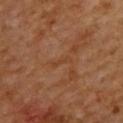This lesion was catalogued during total-body skin photography and was not selected for biopsy. This is a cross-polarized tile. Cropped from a total-body skin-imaging series; the visible field is about 15 mm. The recorded lesion diameter is about 2.5 mm. The subject is a male aged approximately 60. The lesion is on the upper back.